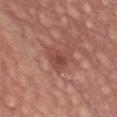The patient is a male roughly 55 years of age. A roughly 15 mm field-of-view crop from a total-body skin photograph. On the front of the torso. Approximately 3 mm at its widest.